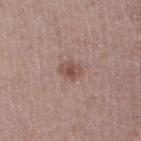Q: What lighting was used for the tile?
A: white-light illumination
Q: What is the lesion's diameter?
A: about 2.5 mm
Q: What kind of image is this?
A: ~15 mm crop, total-body skin-cancer survey
Q: Lesion location?
A: the leg
Q: What are the patient's age and sex?
A: female, aged 48 to 52